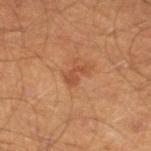| field | value |
|---|---|
| follow-up | total-body-photography surveillance lesion; no biopsy |
| imaging modality | ~15 mm crop, total-body skin-cancer survey |
| illumination | cross-polarized |
| subject | male, aged approximately 45 |
| lesion size | ~2.5 mm (longest diameter) |
| anatomic site | the left lower leg |